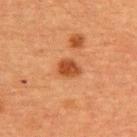Imaged during a routine full-body skin examination; the lesion was not biopsied and no histopathology is available. A close-up tile cropped from a whole-body skin photograph, about 15 mm across. The lesion-visualizer software estimated a lesion color around L≈39 a*≈25 b*≈34 in CIELAB, about 11 CIELAB-L* units darker than the surrounding skin, and a normalized border contrast of about 9. The analysis additionally found a within-lesion color-variation index near 2.5/10 and peripheral color asymmetry of about 1. And it measured a nevus-likeness score of about 95/100. The lesion is located on the upper back. Captured under cross-polarized illumination. A male subject, approximately 50 years of age. Longest diameter approximately 2.5 mm.